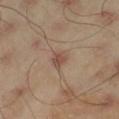No biopsy was performed on this lesion — it was imaged during a full skin examination and was not determined to be concerning. A male subject in their mid- to late 40s. This is a cross-polarized tile. A 15 mm crop from a total-body photograph taken for skin-cancer surveillance. On the left thigh. The recorded lesion diameter is about 3 mm.